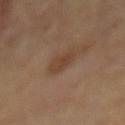biopsy status = total-body-photography surveillance lesion; no biopsy | body site = the back | image source = ~15 mm crop, total-body skin-cancer survey | lesion diameter = ≈3 mm | subject = male, aged 68 to 72 | tile lighting = cross-polarized.